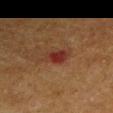<tbp_lesion>
<biopsy_status>not biopsied; imaged during a skin examination</biopsy_status>
<patient>
  <sex>male</sex>
  <age_approx>75</age_approx>
</patient>
<lesion_size>
  <long_diameter_mm_approx>2.5</long_diameter_mm_approx>
</lesion_size>
<image>
  <source>total-body photography crop</source>
  <field_of_view_mm>15</field_of_view_mm>
</image>
<site>right upper arm</site>
<automated_metrics>
  <lesion_detection_confidence_0_100>100</lesion_detection_confidence_0_100>
</automated_metrics>
<lighting>cross-polarized</lighting>
</tbp_lesion>